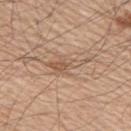| feature | finding |
|---|---|
| biopsy status | no biopsy performed (imaged during a skin exam) |
| image source | total-body-photography crop, ~15 mm field of view |
| diameter | ≈5.5 mm |
| automated metrics | a footprint of about 6.5 mm², a shape eccentricity near 0.9, and two-axis asymmetry of about 0.5; a lesion–skin lightness drop of about 8 and a normalized lesion–skin contrast near 6; internal color variation of about 2 on a 0–10 scale and radial color variation of about 0.5; an automated nevus-likeness rating near 0 out of 100 and a lesion-detection confidence of about 85/100 |
| location | the right upper arm |
| lighting | white-light illumination |
| subject | male, in their mid-50s |